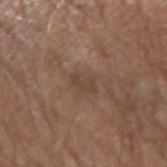Imaged during a routine full-body skin examination; the lesion was not biopsied and no histopathology is available.
The lesion is on the left forearm.
The lesion-visualizer software estimated a shape eccentricity near 0.9 and two-axis asymmetry of about 0.35. The software also gave lesion-presence confidence of about 95/100.
A 15 mm close-up tile from a total-body photography series done for melanoma screening.
Measured at roughly 2.5 mm in maximum diameter.
A male patient roughly 70 years of age.
Imaged with white-light lighting.